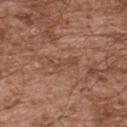The lesion was photographed on a routine skin check and not biopsied; there is no pathology result.
The total-body-photography lesion software estimated an area of roughly 3.5 mm², an outline eccentricity of about 0.95 (0 = round, 1 = elongated), and two-axis asymmetry of about 0.5. It also reported border irregularity of about 6 on a 0–10 scale and radial color variation of about 0. It also reported an automated nevus-likeness rating near 0 out of 100 and a detector confidence of about 85 out of 100 that the crop contains a lesion.
A male patient, roughly 55 years of age.
Imaged with white-light lighting.
The lesion is on the upper back.
Longest diameter approximately 3.5 mm.
This image is a 15 mm lesion crop taken from a total-body photograph.